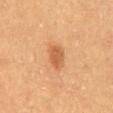biopsy status: catalogued during a skin exam; not biopsied
image source: total-body-photography crop, ~15 mm field of view
diameter: about 3 mm
patient: male, aged 58–62
anatomic site: the abdomen